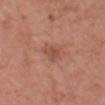Impression:
Captured during whole-body skin photography for melanoma surveillance; the lesion was not biopsied.
Background:
Automated image analysis of the tile measured an outline eccentricity of about 0.7 (0 = round, 1 = elongated) and a symmetry-axis asymmetry near 0.3. It also reported border irregularity of about 3.5 on a 0–10 scale and peripheral color asymmetry of about 1. The analysis additionally found a classifier nevus-likeness of about 0/100 and lesion-presence confidence of about 100/100. The lesion is on the head or neck. A roughly 15 mm field-of-view crop from a total-body skin photograph. The subject is a male aged 58–62. Longest diameter approximately 2.5 mm.